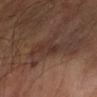Q: Is there a histopathology result?
A: catalogued during a skin exam; not biopsied
Q: Automated lesion metrics?
A: a lesion color around L≈30 a*≈16 b*≈21 in CIELAB and about 5 CIELAB-L* units darker than the surrounding skin; a border-irregularity rating of about 4/10, a color-variation rating of about 3/10, and a peripheral color-asymmetry measure near 1; a nevus-likeness score of about 0/100 and a detector confidence of about 60 out of 100 that the crop contains a lesion
Q: Where on the body is the lesion?
A: the left forearm
Q: What kind of image is this?
A: ~15 mm tile from a whole-body skin photo
Q: Patient demographics?
A: male, roughly 55 years of age
Q: How large is the lesion?
A: ~4 mm (longest diameter)